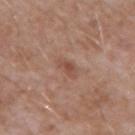Captured during whole-body skin photography for melanoma surveillance; the lesion was not biopsied. Located on the chest. This is a white-light tile. The recorded lesion diameter is about 3 mm. Cropped from a total-body skin-imaging series; the visible field is about 15 mm. The patient is a male about 60 years old.